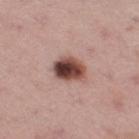The lesion was tiled from a total-body skin photograph and was not biopsied. About 3.5 mm across. On the right thigh. Cropped from a total-body skin-imaging series; the visible field is about 15 mm. The tile uses white-light illumination. A female patient in their mid-30s.